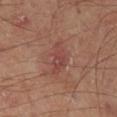Imaged during a routine full-body skin examination; the lesion was not biopsied and no histopathology is available. A 15 mm crop from a total-body photograph taken for skin-cancer surveillance. The patient is a male about 70 years old. The lesion is on the leg. Captured under cross-polarized illumination.